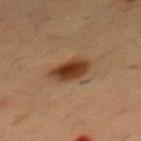biopsy status=total-body-photography surveillance lesion; no biopsy | subject=male, in their mid-50s | anatomic site=the front of the torso | acquisition=~15 mm crop, total-body skin-cancer survey.